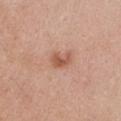Assessment: Part of a total-body skin-imaging series; this lesion was reviewed on a skin check and was not flagged for biopsy. Clinical summary: A region of skin cropped from a whole-body photographic capture, roughly 15 mm wide. A female patient, roughly 25 years of age. The lesion is located on the chest. Measured at roughly 2.5 mm in maximum diameter. The tile uses white-light illumination.